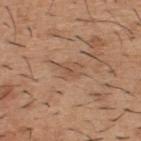Impression: The lesion was photographed on a routine skin check and not biopsied; there is no pathology result. Background: The lesion is located on the upper back. Automated tile analysis of the lesion measured a lesion area of about 5.5 mm², an outline eccentricity of about 0.8 (0 = round, 1 = elongated), and a symmetry-axis asymmetry near 0.35. The analysis additionally found a lesion color around L≈53 a*≈19 b*≈31 in CIELAB, roughly 7 lightness units darker than nearby skin, and a normalized lesion–skin contrast near 5. The software also gave an automated nevus-likeness rating near 0 out of 100. The lesion's longest dimension is about 3.5 mm. A male patient, approximately 40 years of age. Imaged with white-light lighting. A roughly 15 mm field-of-view crop from a total-body skin photograph.